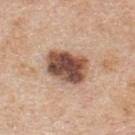<lesion>
  <biopsy_status>not biopsied; imaged during a skin examination</biopsy_status>
  <patient>
    <sex>male</sex>
    <age_approx>60</age_approx>
  </patient>
  <lighting>white-light</lighting>
  <image>
    <source>total-body photography crop</source>
    <field_of_view_mm>15</field_of_view_mm>
  </image>
  <lesion_size>
    <long_diameter_mm_approx>5.0</long_diameter_mm_approx>
  </lesion_size>
  <site>upper back</site>
  <automated_metrics>
    <border_irregularity_0_10>1.5</border_irregularity_0_10>
    <color_variation_0_10>7.0</color_variation_0_10>
    <peripheral_color_asymmetry>2.0</peripheral_color_asymmetry>
  </automated_metrics>
</lesion>